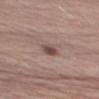Clinical impression: The lesion was photographed on a routine skin check and not biopsied; there is no pathology result. Context: On the right upper arm. A lesion tile, about 15 mm wide, cut from a 3D total-body photograph. The lesion-visualizer software estimated a lesion area of about 4 mm², a shape eccentricity near 0.75, and a symmetry-axis asymmetry near 0.25. A male patient aged 68 to 72.